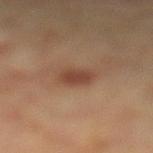Clinical impression:
Imaged during a routine full-body skin examination; the lesion was not biopsied and no histopathology is available.
Context:
A lesion tile, about 15 mm wide, cut from a 3D total-body photograph. Automated tile analysis of the lesion measured an average lesion color of about L≈42 a*≈21 b*≈28 (CIELAB), about 9 CIELAB-L* units darker than the surrounding skin, and a normalized lesion–skin contrast near 7.5. Located on the right leg. A female patient, in their mid- to late 60s. The tile uses cross-polarized illumination.